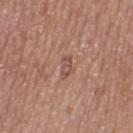Impression:
Imaged during a routine full-body skin examination; the lesion was not biopsied and no histopathology is available.
Image and clinical context:
A roughly 15 mm field-of-view crop from a total-body skin photograph. The lesion is on the left thigh. A female patient, aged 38–42.